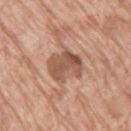biopsy_status: not biopsied; imaged during a skin examination
site: right thigh
patient:
  sex: female
  age_approx: 75
image:
  source: total-body photography crop
  field_of_view_mm: 15